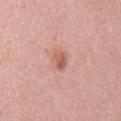Case summary:
* workup — imaged on a skin check; not biopsied
* acquisition — ~15 mm tile from a whole-body skin photo
* subject — female, aged around 70
* lesion diameter — ~2.5 mm (longest diameter)
* TBP lesion metrics — a lesion–skin lightness drop of about 10 and a normalized border contrast of about 7; border irregularity of about 3 on a 0–10 scale, a within-lesion color-variation index near 3.5/10, and a peripheral color-asymmetry measure near 1; a classifier nevus-likeness of about 45/100 and a detector confidence of about 100 out of 100 that the crop contains a lesion
* site — the back